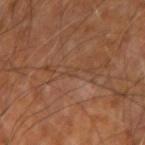• notes: imaged on a skin check; not biopsied
• diameter: ≈1.5 mm
• image source: 15 mm crop, total-body photography
• illumination: cross-polarized illumination
• subject: aged around 65
• body site: the left forearm